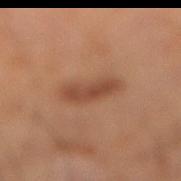biopsy status: catalogued during a skin exam; not biopsied | lighting: cross-polarized | diameter: about 4.5 mm | patient: male, about 60 years old | imaging modality: ~15 mm crop, total-body skin-cancer survey | location: the right lower leg.